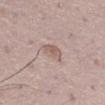Acquisition and patient details:
Longest diameter approximately 3 mm. An algorithmic analysis of the crop reported an area of roughly 6.5 mm². It also reported a lesion-detection confidence of about 100/100. This image is a 15 mm lesion crop taken from a total-body photograph. The lesion is on the left thigh. A male subject, aged approximately 50.